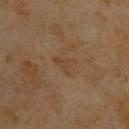biopsy status = total-body-photography surveillance lesion; no biopsy
patient = male, aged 43–47
body site = the upper back
imaging modality = total-body-photography crop, ~15 mm field of view
size = about 3 mm
automated lesion analysis = an area of roughly 4 mm², a shape eccentricity near 0.7, and two-axis asymmetry of about 0.55; a mean CIELAB color near L≈33 a*≈13 b*≈26, roughly 4 lightness units darker than nearby skin, and a normalized lesion–skin contrast near 4.5; internal color variation of about 1.5 on a 0–10 scale and peripheral color asymmetry of about 0.5
illumination = cross-polarized